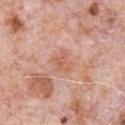Impression: No biopsy was performed on this lesion — it was imaged during a full skin examination and was not determined to be concerning. Acquisition and patient details: Automated image analysis of the tile measured lesion-presence confidence of about 100/100. A male patient, aged 78 to 82. Cropped from a whole-body photographic skin survey; the tile spans about 15 mm. Longest diameter approximately 3 mm. This is a white-light tile. The lesion is on the chest.